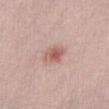| key | value |
|---|---|
| follow-up | no biopsy performed (imaged during a skin exam) |
| image source | 15 mm crop, total-body photography |
| site | the front of the torso |
| size | about 3 mm |
| patient | female, approximately 25 years of age |